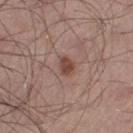The lesion was photographed on a routine skin check and not biopsied; there is no pathology result. This is a white-light tile. About 2.5 mm across. The subject is a male aged around 55. The lesion is located on the left thigh. A close-up tile cropped from a whole-body skin photograph, about 15 mm across.